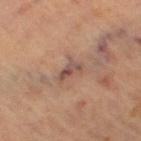{
  "biopsy_status": "not biopsied; imaged during a skin examination"
}